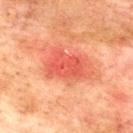Part of a total-body skin-imaging series; this lesion was reviewed on a skin check and was not flagged for biopsy.
A male subject in their mid- to late 70s.
This image is a 15 mm lesion crop taken from a total-body photograph.
On the upper back.